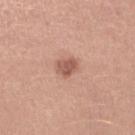biopsy status — catalogued during a skin exam; not biopsied
image-analysis metrics — an average lesion color of about L≈56 a*≈24 b*≈27 (CIELAB), roughly 12 lightness units darker than nearby skin, and a lesion-to-skin contrast of about 7.5 (normalized; higher = more distinct); a border-irregularity rating of about 2.5/10, a within-lesion color-variation index near 2/10, and a peripheral color-asymmetry measure near 0.5
acquisition — ~15 mm crop, total-body skin-cancer survey
illumination — white-light
size — ≈2.5 mm
body site — the left upper arm
patient — female, aged around 35